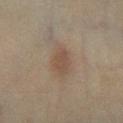follow-up: no biopsy performed (imaged during a skin exam) | site: the right lower leg | subject: female, aged 58 to 62 | imaging modality: ~15 mm tile from a whole-body skin photo.